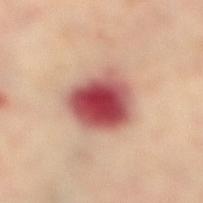Clinical impression:
Part of a total-body skin-imaging series; this lesion was reviewed on a skin check and was not flagged for biopsy.
Acquisition and patient details:
The total-body-photography lesion software estimated an area of roughly 20 mm², an eccentricity of roughly 0.5, and a symmetry-axis asymmetry near 0.2. It also reported roughly 20 lightness units darker than nearby skin. The analysis additionally found an automated nevus-likeness rating near 0 out of 100 and a lesion-detection confidence of about 100/100. The lesion's longest dimension is about 5.5 mm. Imaged with cross-polarized lighting. The patient is a female in their mid-60s. A 15 mm close-up extracted from a 3D total-body photography capture. The lesion is located on the right lower leg.